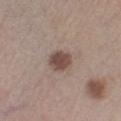Clinical impression: No biopsy was performed on this lesion — it was imaged during a full skin examination and was not determined to be concerning. Image and clinical context: A 15 mm crop from a total-body photograph taken for skin-cancer surveillance. The lesion's longest dimension is about 3 mm. This is a white-light tile. Automated tile analysis of the lesion measured a mean CIELAB color near L≈47 a*≈16 b*≈22 and a lesion-to-skin contrast of about 9.5 (normalized; higher = more distinct). The software also gave border irregularity of about 1.5 on a 0–10 scale, a within-lesion color-variation index near 3/10, and a peripheral color-asymmetry measure near 1. The analysis additionally found a detector confidence of about 100 out of 100 that the crop contains a lesion. From the leg. A male subject, in their mid-50s.